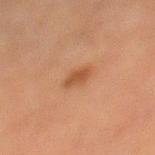{"biopsy_status": "not biopsied; imaged during a skin examination", "site": "left lower leg", "lighting": "cross-polarized", "patient": {"sex": "female", "age_approx": 60}, "image": {"source": "total-body photography crop", "field_of_view_mm": 15}, "lesion_size": {"long_diameter_mm_approx": 2.5}, "automated_metrics": {"vs_skin_darker_L": 8.0, "vs_skin_contrast_norm": 7.0}}